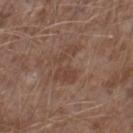biopsy status: total-body-photography surveillance lesion; no biopsy
lesion size: about 5 mm
automated metrics: a lesion area of about 7.5 mm², an eccentricity of roughly 0.85, and a symmetry-axis asymmetry near 0.65; a mean CIELAB color near L≈42 a*≈18 b*≈26, roughly 6 lightness units darker than nearby skin, and a normalized border contrast of about 5.5
illumination: white-light
body site: the leg
image source: total-body-photography crop, ~15 mm field of view
patient: male, approximately 45 years of age